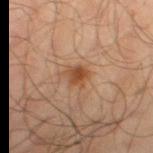Case summary:
– anatomic site — the right thigh
– acquisition — ~15 mm tile from a whole-body skin photo
– tile lighting — cross-polarized
– size — ≈3.5 mm
– patient — male, in their mid- to late 60s